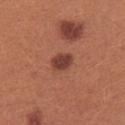<record>
<biopsy_status>not biopsied; imaged during a skin examination</biopsy_status>
<patient>
  <sex>female</sex>
  <age_approx>25</age_approx>
</patient>
<site>right forearm</site>
<lesion_size>
  <long_diameter_mm_approx>2.5</long_diameter_mm_approx>
</lesion_size>
<lighting>white-light</lighting>
<image>
  <source>total-body photography crop</source>
  <field_of_view_mm>15</field_of_view_mm>
</image>
</record>